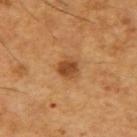biopsy status = no biopsy performed (imaged during a skin exam)
patient = male, in their mid- to late 60s
body site = the right upper arm
image = ~15 mm crop, total-body skin-cancer survey